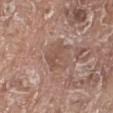{
  "biopsy_status": "not biopsied; imaged during a skin examination",
  "image": {
    "source": "total-body photography crop",
    "field_of_view_mm": 15
  },
  "patient": {
    "sex": "male",
    "age_approx": 75
  },
  "site": "leg"
}